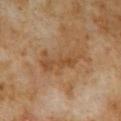{
  "biopsy_status": "not biopsied; imaged during a skin examination",
  "automated_metrics": {
    "area_mm2_approx": 11.0,
    "eccentricity": 0.9,
    "shape_asymmetry": 0.4,
    "vs_skin_darker_L": 7.0,
    "vs_skin_contrast_norm": 6.0,
    "border_irregularity_0_10": 6.0,
    "color_variation_0_10": 3.5,
    "peripheral_color_asymmetry": 1.0,
    "nevus_likeness_0_100": 0,
    "lesion_detection_confidence_0_100": 100
  },
  "site": "abdomen",
  "image": {
    "source": "total-body photography crop",
    "field_of_view_mm": 15
  },
  "lesion_size": {
    "long_diameter_mm_approx": 5.5
  },
  "lighting": "cross-polarized",
  "patient": {
    "sex": "male",
    "age_approx": 60
  }
}